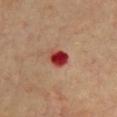Q: Was a biopsy performed?
A: catalogued during a skin exam; not biopsied
Q: What is the lesion's diameter?
A: ≈2.5 mm
Q: Where on the body is the lesion?
A: the chest
Q: How was the tile lit?
A: cross-polarized
Q: Automated lesion metrics?
A: a lesion area of about 5 mm², an outline eccentricity of about 0.5 (0 = round, 1 = elongated), and a shape-asymmetry score of about 0.25 (0 = symmetric); border irregularity of about 2 on a 0–10 scale and a peripheral color-asymmetry measure near 1.5
Q: Who is the patient?
A: aged approximately 60
Q: How was this image acquired?
A: total-body-photography crop, ~15 mm field of view